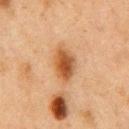Background:
The lesion's longest dimension is about 4.5 mm. Imaged with cross-polarized lighting. An algorithmic analysis of the crop reported a mean CIELAB color near L≈44 a*≈20 b*≈34, roughly 11 lightness units darker than nearby skin, and a normalized border contrast of about 9.5. It also reported a classifier nevus-likeness of about 95/100 and a detector confidence of about 100 out of 100 that the crop contains a lesion. Located on the chest. A male patient, roughly 75 years of age. A roughly 15 mm field-of-view crop from a total-body skin photograph.